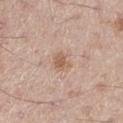Clinical impression:
No biopsy was performed on this lesion — it was imaged during a full skin examination and was not determined to be concerning.
Image and clinical context:
Located on the right thigh. The subject is a male in their mid- to late 60s. A 15 mm close-up extracted from a 3D total-body photography capture.